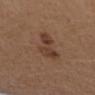Clinical impression: Captured during whole-body skin photography for melanoma surveillance; the lesion was not biopsied. Image and clinical context: The tile uses white-light illumination. Automated tile analysis of the lesion measured an outline eccentricity of about 0.85 (0 = round, 1 = elongated). And it measured an average lesion color of about L≈39 a*≈18 b*≈27 (CIELAB), a lesion–skin lightness drop of about 9, and a normalized border contrast of about 7.5. The software also gave a color-variation rating of about 3.5/10 and radial color variation of about 1. The subject is a female approximately 40 years of age. Approximately 4.5 mm at its widest. Located on the left forearm. A close-up tile cropped from a whole-body skin photograph, about 15 mm across.